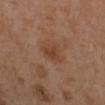{
  "biopsy_status": "not biopsied; imaged during a skin examination",
  "patient": {
    "sex": "female",
    "age_approx": 40
  },
  "image": {
    "source": "total-body photography crop",
    "field_of_view_mm": 15
  },
  "site": "left arm"
}